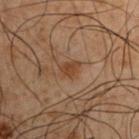Captured during whole-body skin photography for melanoma surveillance; the lesion was not biopsied.
The lesion's longest dimension is about 2.5 mm.
The lesion is on the right upper arm.
This is a cross-polarized tile.
A male patient, roughly 50 years of age.
A 15 mm close-up extracted from a 3D total-body photography capture.